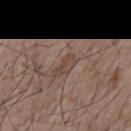Recorded during total-body skin imaging; not selected for excision or biopsy. A region of skin cropped from a whole-body photographic capture, roughly 15 mm wide. The lesion's longest dimension is about 3.5 mm. This is a white-light tile. Located on the front of the torso. The patient is a male approximately 75 years of age.